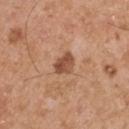No biopsy was performed on this lesion — it was imaged during a full skin examination and was not determined to be concerning. A roughly 15 mm field-of-view crop from a total-body skin photograph. The tile uses white-light illumination. A male patient in their mid-50s. On the right upper arm. Longest diameter approximately 3 mm.